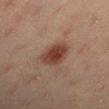Captured during whole-body skin photography for melanoma surveillance; the lesion was not biopsied. About 3.5 mm across. The lesion is located on the left lower leg. The patient is a female aged approximately 20. A roughly 15 mm field-of-view crop from a total-body skin photograph. The total-body-photography lesion software estimated an outline eccentricity of about 0.4 (0 = round, 1 = elongated) and a symmetry-axis asymmetry near 0.2. It also reported a lesion color around L≈35 a*≈19 b*≈24 in CIELAB and a normalized lesion–skin contrast near 10. And it measured an automated nevus-likeness rating near 100 out of 100 and lesion-presence confidence of about 100/100.